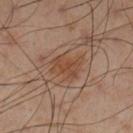Q: Was this lesion biopsied?
A: total-body-photography surveillance lesion; no biopsy
Q: Lesion location?
A: the left lower leg
Q: What did automated image analysis measure?
A: about 7 CIELAB-L* units darker than the surrounding skin and a lesion-to-skin contrast of about 6.5 (normalized; higher = more distinct); an automated nevus-likeness rating near 25 out of 100 and lesion-presence confidence of about 100/100
Q: What is the imaging modality?
A: 15 mm crop, total-body photography
Q: What are the patient's age and sex?
A: male, aged 53–57